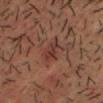Assessment:
Imaged during a routine full-body skin examination; the lesion was not biopsied and no histopathology is available.
Acquisition and patient details:
A 15 mm close-up tile from a total-body photography series done for melanoma screening. A male patient approximately 35 years of age. On the head or neck. The recorded lesion diameter is about 3.5 mm.